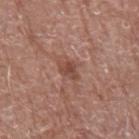{"biopsy_status": "not biopsied; imaged during a skin examination", "site": "left upper arm", "automated_metrics": {"area_mm2_approx": 4.0, "eccentricity": 0.7, "shape_asymmetry": 0.3, "border_irregularity_0_10": 2.5, "color_variation_0_10": 3.0, "peripheral_color_asymmetry": 1.0}, "patient": {"sex": "male", "age_approx": 70}, "lighting": "white-light", "image": {"source": "total-body photography crop", "field_of_view_mm": 15}}